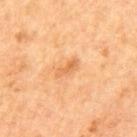follow-up — total-body-photography surveillance lesion; no biopsy.